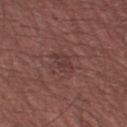Impression: Captured during whole-body skin photography for melanoma surveillance; the lesion was not biopsied. Clinical summary: An algorithmic analysis of the crop reported a footprint of about 4 mm² and a shape-asymmetry score of about 0.25 (0 = symmetric). A 15 mm close-up tile from a total-body photography series done for melanoma screening. Longest diameter approximately 2.5 mm. The lesion is on the right thigh. The subject is a male in their mid-40s. Imaged with white-light lighting.